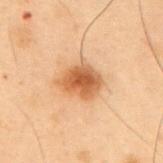  biopsy_status: not biopsied; imaged during a skin examination
  lighting: cross-polarized
  image:
    source: total-body photography crop
    field_of_view_mm: 15
  site: back
  patient:
    sex: male
    age_approx: 55